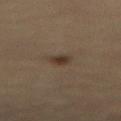| key | value |
|---|---|
| notes | total-body-photography surveillance lesion; no biopsy |
| acquisition | 15 mm crop, total-body photography |
| site | the front of the torso |
| lesion size | ~2 mm (longest diameter) |
| tile lighting | cross-polarized |
| subject | female, in their mid-60s |